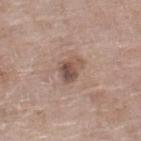Measured at roughly 3 mm in maximum diameter. A roughly 15 mm field-of-view crop from a total-body skin photograph. From the right lower leg. The patient is a female aged 73–77. Automated image analysis of the tile measured an average lesion color of about L≈50 a*≈17 b*≈24 (CIELAB). The analysis additionally found a border-irregularity index near 5/10, internal color variation of about 4 on a 0–10 scale, and peripheral color asymmetry of about 1. The software also gave a classifier nevus-likeness of about 30/100 and a lesion-detection confidence of about 100/100.